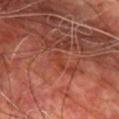Captured during whole-body skin photography for melanoma surveillance; the lesion was not biopsied.
Automated image analysis of the tile measured an outline eccentricity of about 0.95 (0 = round, 1 = elongated) and two-axis asymmetry of about 0.35. It also reported a color-variation rating of about 0/10 and peripheral color asymmetry of about 0.
A 15 mm close-up extracted from a 3D total-body photography capture.
From the front of the torso.
A male patient, approximately 60 years of age.
The lesion's longest dimension is about 2.5 mm.
Captured under cross-polarized illumination.